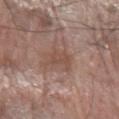Clinical impression: The lesion was photographed on a routine skin check and not biopsied; there is no pathology result. Context: The subject is a male in their mid- to late 60s. A roughly 15 mm field-of-view crop from a total-body skin photograph. The tile uses white-light illumination. Automated image analysis of the tile measured a lesion area of about 5 mm², a shape eccentricity near 0.65, and a symmetry-axis asymmetry near 0.35. The analysis additionally found a lesion-to-skin contrast of about 5.5 (normalized; higher = more distinct). From the left forearm. The recorded lesion diameter is about 3 mm.